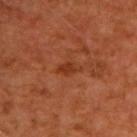biopsy status: catalogued during a skin exam; not biopsied | imaging modality: ~15 mm tile from a whole-body skin photo | lesion diameter: ~3 mm (longest diameter) | subject: male, aged around 65 | body site: the upper back | lighting: cross-polarized illumination.